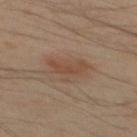lighting: cross-polarized illumination; location: the mid back; lesion size: ~4 mm (longest diameter); acquisition: ~15 mm tile from a whole-body skin photo; subject: male, roughly 50 years of age.